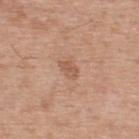No biopsy was performed on this lesion — it was imaged during a full skin examination and was not determined to be concerning. A male patient in their mid-50s. A 15 mm close-up extracted from a 3D total-body photography capture. Measured at roughly 2.5 mm in maximum diameter. An algorithmic analysis of the crop reported a lesion area of about 3 mm² and a symmetry-axis asymmetry near 0.25. The lesion is located on the upper back.